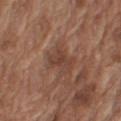This lesion was catalogued during total-body skin photography and was not selected for biopsy. Imaged with white-light lighting. Located on the right upper arm. Automated tile analysis of the lesion measured an average lesion color of about L≈41 a*≈20 b*≈27 (CIELAB), roughly 7 lightness units darker than nearby skin, and a normalized border contrast of about 6. It also reported border irregularity of about 4 on a 0–10 scale and internal color variation of about 3 on a 0–10 scale. The software also gave a classifier nevus-likeness of about 0/100 and a detector confidence of about 95 out of 100 that the crop contains a lesion. The subject is a male aged around 75. Longest diameter approximately 3.5 mm. A lesion tile, about 15 mm wide, cut from a 3D total-body photograph.